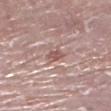workup — imaged on a skin check; not biopsied | illumination — white-light illumination | image source — 15 mm crop, total-body photography | anatomic site — the leg | lesion diameter — about 2.5 mm | patient — female, aged around 75.